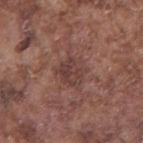Impression: The lesion was photographed on a routine skin check and not biopsied; there is no pathology result. Acquisition and patient details: Located on the arm. A male patient, aged 73–77. A region of skin cropped from a whole-body photographic capture, roughly 15 mm wide. Approximately 3.5 mm at its widest. Automated tile analysis of the lesion measured a lesion color around L≈40 a*≈20 b*≈22 in CIELAB, a lesion–skin lightness drop of about 7, and a normalized lesion–skin contrast near 6. The software also gave a border-irregularity rating of about 2.5/10, a color-variation rating of about 3.5/10, and a peripheral color-asymmetry measure near 1.5. The software also gave an automated nevus-likeness rating near 0 out of 100.